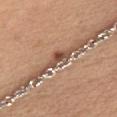Findings:
– size — ≈3 mm
– subject — female, aged 43 to 47
– anatomic site — the chest
– lighting — white-light illumination
– image source — ~15 mm crop, total-body skin-cancer survey